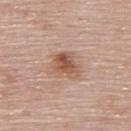workup: no biopsy performed (imaged during a skin exam)
image source: ~15 mm crop, total-body skin-cancer survey
subject: female, in their mid- to late 60s
automated lesion analysis: a footprint of about 9.5 mm², a shape eccentricity near 0.6, and a shape-asymmetry score of about 0.25 (0 = symmetric); a mean CIELAB color near L≈56 a*≈21 b*≈29 and a lesion–skin lightness drop of about 10; an automated nevus-likeness rating near 90 out of 100 and a lesion-detection confidence of about 100/100
lesion size: ≈4 mm
lighting: white-light
site: the upper back